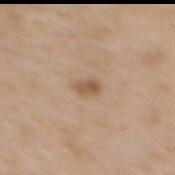| key | value |
|---|---|
| patient | female, aged around 65 |
| tile lighting | white-light illumination |
| imaging modality | ~15 mm crop, total-body skin-cancer survey |
| site | the upper back |